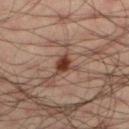| feature | finding |
|---|---|
| biopsy status | catalogued during a skin exam; not biopsied |
| diameter | about 3 mm |
| imaging modality | ~15 mm crop, total-body skin-cancer survey |
| illumination | cross-polarized |
| subject | male, aged 33–37 |
| image-analysis metrics | an area of roughly 4 mm² and two-axis asymmetry of about 0.45; a border-irregularity rating of about 5/10, internal color variation of about 3 on a 0–10 scale, and a peripheral color-asymmetry measure near 1; a detector confidence of about 100 out of 100 that the crop contains a lesion |
| body site | the left lower leg |